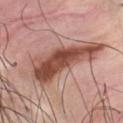The lesion was photographed on a routine skin check and not biopsied; there is no pathology result. The subject is a male approximately 45 years of age. The tile uses white-light illumination. Located on the chest. Measured at roughly 10 mm in maximum diameter. Automated tile analysis of the lesion measured a mean CIELAB color near L≈50 a*≈24 b*≈27, roughly 16 lightness units darker than nearby skin, and a normalized lesion–skin contrast near 10.5. It also reported a border-irregularity rating of about 5.5/10. A region of skin cropped from a whole-body photographic capture, roughly 15 mm wide.